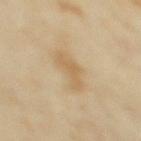No biopsy was performed on this lesion — it was imaged during a full skin examination and was not determined to be concerning. Cropped from a total-body skin-imaging series; the visible field is about 15 mm. Automated tile analysis of the lesion measured a footprint of about 8 mm², an outline eccentricity of about 0.8 (0 = round, 1 = elongated), and a symmetry-axis asymmetry near 0.3. The analysis additionally found a border-irregularity index near 4/10, a within-lesion color-variation index near 2/10, and a peripheral color-asymmetry measure near 0.5. The patient is a female roughly 35 years of age. Longest diameter approximately 4 mm. On the upper back. The tile uses cross-polarized illumination.